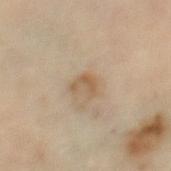Q: Was a biopsy performed?
A: imaged on a skin check; not biopsied
Q: What did automated image analysis measure?
A: a footprint of about 4 mm², an eccentricity of roughly 0.7, and a symmetry-axis asymmetry near 0.4; internal color variation of about 2 on a 0–10 scale and radial color variation of about 0.5
Q: Lesion size?
A: ~2.5 mm (longest diameter)
Q: What are the patient's age and sex?
A: female, about 60 years old
Q: How was this image acquired?
A: total-body-photography crop, ~15 mm field of view
Q: Where on the body is the lesion?
A: the left thigh